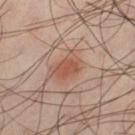Imaged during a routine full-body skin examination; the lesion was not biopsied and no histopathology is available. On the left thigh. Longest diameter approximately 3.5 mm. The patient is a male aged around 40. A close-up tile cropped from a whole-body skin photograph, about 15 mm across.